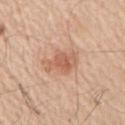biopsy_status: not biopsied; imaged during a skin examination
patient:
  sex: male
  age_approx: 55
image:
  source: total-body photography crop
  field_of_view_mm: 15
lighting: white-light
site: right upper arm
automated_metrics:
  area_mm2_approx: 8.5
  eccentricity: 0.6
  shape_asymmetry: 0.25
  cielab_L: 61
  cielab_a: 22
  cielab_b: 32
  vs_skin_darker_L: 10.0
  vs_skin_contrast_norm: 6.5
  border_irregularity_0_10: 3.0
  color_variation_0_10: 4.0
  peripheral_color_asymmetry: 1.0